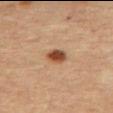Assessment: No biopsy was performed on this lesion — it was imaged during a full skin examination and was not determined to be concerning. Clinical summary: The lesion is located on the abdomen. The subject is a male about 65 years old. Automated tile analysis of the lesion measured an area of roughly 4.5 mm², an eccentricity of roughly 0.5, and two-axis asymmetry of about 0.2. The analysis additionally found a classifier nevus-likeness of about 100/100 and a detector confidence of about 100 out of 100 that the crop contains a lesion. A roughly 15 mm field-of-view crop from a total-body skin photograph. Approximately 2.5 mm at its widest. Captured under cross-polarized illumination.